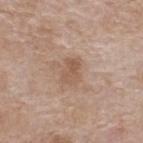Q: Was a biopsy performed?
A: no biopsy performed (imaged during a skin exam)
Q: How was this image acquired?
A: ~15 mm tile from a whole-body skin photo
Q: Lesion location?
A: the upper back
Q: Patient demographics?
A: female, about 75 years old